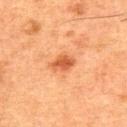notes: total-body-photography surveillance lesion; no biopsy
lesion size: ~3 mm (longest diameter)
tile lighting: cross-polarized illumination
body site: the upper back
subject: male, aged approximately 50
imaging modality: total-body-photography crop, ~15 mm field of view